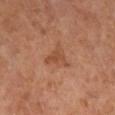Impression:
Imaged during a routine full-body skin examination; the lesion was not biopsied and no histopathology is available.